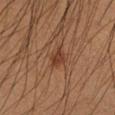The lesion was photographed on a routine skin check and not biopsied; there is no pathology result. The recorded lesion diameter is about 3 mm. This image is a 15 mm lesion crop taken from a total-body photograph. On the left forearm. The patient is a male about 35 years old. Captured under cross-polarized illumination.